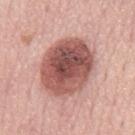<tbp_lesion>
<lesion_size>
  <long_diameter_mm_approx>7.5</long_diameter_mm_approx>
</lesion_size>
<patient>
  <sex>male</sex>
  <age_approx>75</age_approx>
</patient>
<image>
  <source>total-body photography crop</source>
  <field_of_view_mm>15</field_of_view_mm>
</image>
<site>mid back</site>
<lighting>white-light</lighting>
</tbp_lesion>